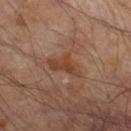This lesion was catalogued during total-body skin photography and was not selected for biopsy.
Cropped from a whole-body photographic skin survey; the tile spans about 15 mm.
A male subject, aged approximately 65.
Automated image analysis of the tile measured a footprint of about 7 mm². The analysis additionally found a border-irregularity index near 5/10, internal color variation of about 2.5 on a 0–10 scale, and a peripheral color-asymmetry measure near 1. It also reported a nevus-likeness score of about 0/100 and a detector confidence of about 100 out of 100 that the crop contains a lesion.
Located on the right lower leg.
Measured at roughly 4.5 mm in maximum diameter.
Captured under cross-polarized illumination.